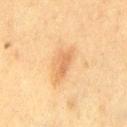Part of a total-body skin-imaging series; this lesion was reviewed on a skin check and was not flagged for biopsy. From the left thigh. A 15 mm crop from a total-body photograph taken for skin-cancer surveillance. The lesion's longest dimension is about 4 mm. A female subject aged 53 to 57. The tile uses cross-polarized illumination.